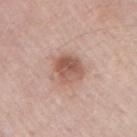Case summary:
• workup · imaged on a skin check; not biopsied
• body site · the right upper arm
• illumination · white-light illumination
• size · ~3.5 mm (longest diameter)
• patient · male, aged approximately 55
• acquisition · ~15 mm crop, total-body skin-cancer survey
• automated metrics · a lesion area of about 9.5 mm², a shape eccentricity near 0.45, and a shape-asymmetry score of about 0.2 (0 = symmetric); a lesion–skin lightness drop of about 12 and a lesion-to-skin contrast of about 8 (normalized; higher = more distinct); a within-lesion color-variation index near 6/10; a nevus-likeness score of about 85/100 and a lesion-detection confidence of about 100/100